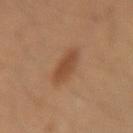<tbp_lesion>
<biopsy_status>not biopsied; imaged during a skin examination</biopsy_status>
<site>arm</site>
<patient>
  <sex>female</sex>
  <age_approx>35</age_approx>
</patient>
<image>
  <source>total-body photography crop</source>
  <field_of_view_mm>15</field_of_view_mm>
</image>
</tbp_lesion>